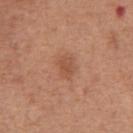Imaged during a routine full-body skin examination; the lesion was not biopsied and no histopathology is available. The recorded lesion diameter is about 2.5 mm. On the abdomen. A male patient roughly 65 years of age. Captured under white-light illumination. Cropped from a total-body skin-imaging series; the visible field is about 15 mm.